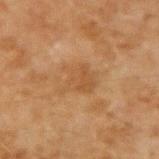<tbp_lesion>
  <biopsy_status>not biopsied; imaged during a skin examination</biopsy_status>
  <patient>
    <sex>male</sex>
    <age_approx>45</age_approx>
  </patient>
  <image>
    <source>total-body photography crop</source>
    <field_of_view_mm>15</field_of_view_mm>
  </image>
  <automated_metrics>
    <area_mm2_approx>9.0</area_mm2_approx>
    <eccentricity>0.6</eccentricity>
    <shape_asymmetry>0.45</shape_asymmetry>
    <cielab_L>42</cielab_L>
    <cielab_a>17</cielab_a>
    <cielab_b>32</cielab_b>
    <vs_skin_darker_L>5.0</vs_skin_darker_L>
    <vs_skin_contrast_norm>5.0</vs_skin_contrast_norm>
    <nevus_likeness_0_100>0</nevus_likeness_0_100>
    <lesion_detection_confidence_0_100>100</lesion_detection_confidence_0_100>
  </automated_metrics>
  <lighting>cross-polarized</lighting>
  <site>right upper arm</site>
</tbp_lesion>